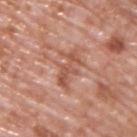Part of a total-body skin-imaging series; this lesion was reviewed on a skin check and was not flagged for biopsy.
The lesion is on the upper back.
A male patient, roughly 70 years of age.
Imaged with white-light lighting.
A lesion tile, about 15 mm wide, cut from a 3D total-body photograph.
An algorithmic analysis of the crop reported border irregularity of about 8 on a 0–10 scale, internal color variation of about 2 on a 0–10 scale, and peripheral color asymmetry of about 0.
Longest diameter approximately 4.5 mm.